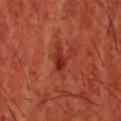Assessment:
Recorded during total-body skin imaging; not selected for excision or biopsy.
Acquisition and patient details:
The lesion is located on the head or neck. A male patient aged approximately 45. Longest diameter approximately 3 mm. This image is a 15 mm lesion crop taken from a total-body photograph. The tile uses cross-polarized illumination.